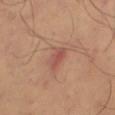Imaged during a routine full-body skin examination; the lesion was not biopsied and no histopathology is available.
Automated tile analysis of the lesion measured an area of roughly 3.5 mm², an eccentricity of roughly 0.9, and a shape-asymmetry score of about 0.4 (0 = symmetric). The analysis additionally found a lesion color around L≈52 a*≈26 b*≈27 in CIELAB and a normalized lesion–skin contrast near 6.5. And it measured border irregularity of about 3.5 on a 0–10 scale and a peripheral color-asymmetry measure near 0.5.
The lesion is located on the right thigh.
This is a cross-polarized tile.
A roughly 15 mm field-of-view crop from a total-body skin photograph.
The recorded lesion diameter is about 3 mm.